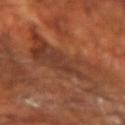Part of a total-body skin-imaging series; this lesion was reviewed on a skin check and was not flagged for biopsy.
A male patient approximately 70 years of age.
Cropped from a total-body skin-imaging series; the visible field is about 15 mm.
On the left forearm.